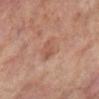{
  "biopsy_status": "not biopsied; imaged during a skin examination",
  "automated_metrics": {
    "area_mm2_approx": 7.5,
    "eccentricity": 0.6,
    "cielab_L": 53,
    "cielab_a": 21,
    "cielab_b": 28,
    "vs_skin_darker_L": 6.0,
    "nevus_likeness_0_100": 5,
    "lesion_detection_confidence_0_100": 100
  },
  "patient": {
    "sex": "male",
    "age_approx": 70
  },
  "site": "right lower leg",
  "lesion_size": {
    "long_diameter_mm_approx": 3.5
  },
  "lighting": "cross-polarized",
  "image": {
    "source": "total-body photography crop",
    "field_of_view_mm": 15
  }
}